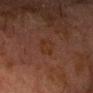A male patient, roughly 75 years of age. A 15 mm close-up extracted from a 3D total-body photography capture. The lesion is on the chest. The recorded lesion diameter is about 3 mm.